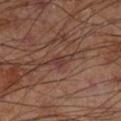The lesion was tiled from a total-body skin photograph and was not biopsied.